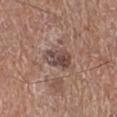biopsy status = no biopsy performed (imaged during a skin exam).